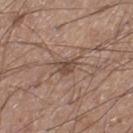Assessment:
Part of a total-body skin-imaging series; this lesion was reviewed on a skin check and was not flagged for biopsy.
Image and clinical context:
The total-body-photography lesion software estimated a lesion area of about 4 mm², an outline eccentricity of about 0.7 (0 = round, 1 = elongated), and a shape-asymmetry score of about 0.35 (0 = symmetric). The software also gave a mean CIELAB color near L≈47 a*≈17 b*≈25, roughly 9 lightness units darker than nearby skin, and a normalized lesion–skin contrast near 7. The analysis additionally found a border-irregularity index near 3.5/10, internal color variation of about 3 on a 0–10 scale, and radial color variation of about 1. And it measured an automated nevus-likeness rating near 0 out of 100. Measured at roughly 2.5 mm in maximum diameter. From the right lower leg. A male subject aged 18 to 22. Cropped from a whole-body photographic skin survey; the tile spans about 15 mm. The tile uses white-light illumination.